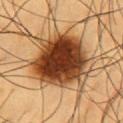Q: Is there a histopathology result?
A: catalogued during a skin exam; not biopsied
Q: How was this image acquired?
A: 15 mm crop, total-body photography
Q: What are the patient's age and sex?
A: male, aged approximately 60
Q: What is the lesion's diameter?
A: ~7.5 mm (longest diameter)
Q: Lesion location?
A: the chest